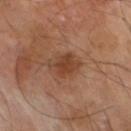follow-up — no biopsy performed (imaged during a skin exam)
image — ~15 mm crop, total-body skin-cancer survey
subject — male, aged 68–72
anatomic site — the right thigh
size — ≈3.5 mm
TBP lesion metrics — a footprint of about 6.5 mm², an eccentricity of roughly 0.45, and two-axis asymmetry of about 0.2; a lesion color around L≈40 a*≈22 b*≈31 in CIELAB, roughly 9 lightness units darker than nearby skin, and a lesion-to-skin contrast of about 7.5 (normalized; higher = more distinct); a border-irregularity index near 2.5/10, internal color variation of about 2.5 on a 0–10 scale, and a peripheral color-asymmetry measure near 1; lesion-presence confidence of about 100/100
lighting — cross-polarized